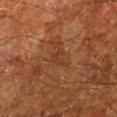The lesion was tiled from a total-body skin photograph and was not biopsied.